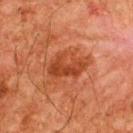{
  "biopsy_status": "not biopsied; imaged during a skin examination",
  "image": {
    "source": "total-body photography crop",
    "field_of_view_mm": 15
  },
  "lighting": "cross-polarized",
  "automated_metrics": {
    "border_irregularity_0_10": 4.5,
    "color_variation_0_10": 4.5,
    "peripheral_color_asymmetry": 2.0,
    "nevus_likeness_0_100": 35,
    "lesion_detection_confidence_0_100": 100
  },
  "patient": {
    "sex": "male",
    "age_approx": 65
  },
  "site": "upper back",
  "lesion_size": {
    "long_diameter_mm_approx": 5.0
  }
}